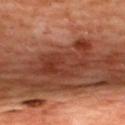Part of a total-body skin-imaging series; this lesion was reviewed on a skin check and was not flagged for biopsy. The recorded lesion diameter is about 7 mm. A female patient in their mid-40s. A close-up tile cropped from a whole-body skin photograph, about 15 mm across. On the upper back.